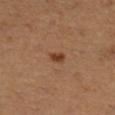biopsy_status: not biopsied; imaged during a skin examination
patient:
  sex: female
  age_approx: 55
lesion_size:
  long_diameter_mm_approx: 2.0
site: right lower leg
image:
  source: total-body photography crop
  field_of_view_mm: 15
lighting: cross-polarized